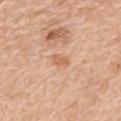biopsy_status: not biopsied; imaged during a skin examination
patient:
  sex: female
  age_approx: 65
site: mid back
image:
  source: total-body photography crop
  field_of_view_mm: 15